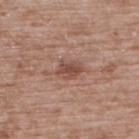Captured during whole-body skin photography for melanoma surveillance; the lesion was not biopsied.
A male patient aged approximately 50.
Cropped from a total-body skin-imaging series; the visible field is about 15 mm.
On the upper back.
The recorded lesion diameter is about 3 mm.